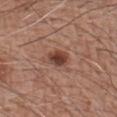Q: Is there a histopathology result?
A: total-body-photography surveillance lesion; no biopsy
Q: Illumination type?
A: white-light
Q: What did automated image analysis measure?
A: a lesion–skin lightness drop of about 12; a classifier nevus-likeness of about 95/100
Q: What is the imaging modality?
A: ~15 mm crop, total-body skin-cancer survey
Q: What is the anatomic site?
A: the left forearm
Q: Who is the patient?
A: male, aged 58–62
Q: How large is the lesion?
A: about 2.5 mm